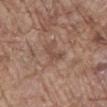<case>
<biopsy_status>not biopsied; imaged during a skin examination</biopsy_status>
<image>
  <source>total-body photography crop</source>
  <field_of_view_mm>15</field_of_view_mm>
</image>
<patient>
  <sex>male</sex>
  <age_approx>80</age_approx>
</patient>
<site>abdomen</site>
</case>